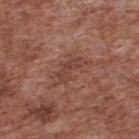biopsy status: total-body-photography surveillance lesion; no biopsy
tile lighting: white-light illumination
TBP lesion metrics: a lesion color around L≈43 a*≈22 b*≈27 in CIELAB and roughly 7 lightness units darker than nearby skin; border irregularity of about 5.5 on a 0–10 scale and internal color variation of about 1.5 on a 0–10 scale; a classifier nevus-likeness of about 0/100 and a lesion-detection confidence of about 100/100
imaging modality: 15 mm crop, total-body photography
site: the upper back
lesion diameter: ~4.5 mm (longest diameter)
subject: male, approximately 75 years of age